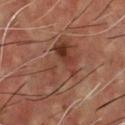Q: Was a biopsy performed?
A: no biopsy performed (imaged during a skin exam)
Q: What is the imaging modality?
A: ~15 mm tile from a whole-body skin photo
Q: What is the anatomic site?
A: the chest
Q: Patient demographics?
A: male, aged 53 to 57
Q: How large is the lesion?
A: ~7 mm (longest diameter)
Q: Illumination type?
A: cross-polarized